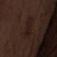No biopsy was performed on this lesion — it was imaged during a full skin examination and was not determined to be concerning. Located on the abdomen. This is a white-light tile. About 4.5 mm across. A male patient about 70 years old. Cropped from a whole-body photographic skin survey; the tile spans about 15 mm.